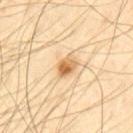Part of a total-body skin-imaging series; this lesion was reviewed on a skin check and was not flagged for biopsy. This image is a 15 mm lesion crop taken from a total-body photograph. From the mid back. The tile uses cross-polarized illumination. The patient is a male aged around 65.